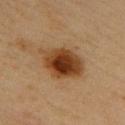No biopsy was performed on this lesion — it was imaged during a full skin examination and was not determined to be concerning. Located on the upper back. A lesion tile, about 15 mm wide, cut from a 3D total-body photograph. This is a cross-polarized tile. A female patient aged approximately 45. An algorithmic analysis of the crop reported a lesion area of about 16 mm². The analysis additionally found a lesion color around L≈34 a*≈19 b*≈32 in CIELAB and about 14 CIELAB-L* units darker than the surrounding skin. The software also gave a nevus-likeness score of about 100/100 and lesion-presence confidence of about 100/100.